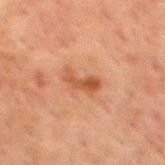Impression: The lesion was photographed on a routine skin check and not biopsied; there is no pathology result. Context: Automated image analysis of the tile measured a border-irregularity rating of about 4.5/10 and peripheral color asymmetry of about 0.5. The software also gave a classifier nevus-likeness of about 0/100 and a lesion-detection confidence of about 100/100. A region of skin cropped from a whole-body photographic capture, roughly 15 mm wide. Longest diameter approximately 3.5 mm. From the mid back. The tile uses cross-polarized illumination. A male patient approximately 60 years of age.